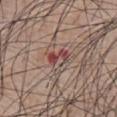Captured during whole-body skin photography for melanoma surveillance; the lesion was not biopsied. A male patient in their mid-70s. About 3 mm across. A 15 mm close-up tile from a total-body photography series done for melanoma screening. On the abdomen. This is a white-light tile. The lesion-visualizer software estimated a lesion color around L≈46 a*≈23 b*≈21 in CIELAB, about 10 CIELAB-L* units darker than the surrounding skin, and a lesion-to-skin contrast of about 8 (normalized; higher = more distinct). And it measured a classifier nevus-likeness of about 0/100 and lesion-presence confidence of about 100/100.